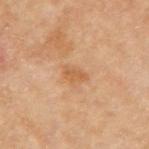– workup · catalogued during a skin exam; not biopsied
– lighting · cross-polarized illumination
– body site · the right upper arm
– image source · ~15 mm tile from a whole-body skin photo
– size · ~3 mm (longest diameter)
– subject · female, aged 58 to 62